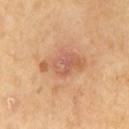Case summary:
* workup: total-body-photography surveillance lesion; no biopsy